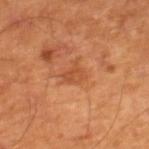tile lighting=cross-polarized | acquisition=15 mm crop, total-body photography | patient=male, roughly 65 years of age | lesion diameter=about 2.5 mm.